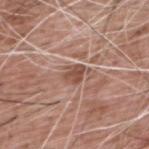Case summary:
– notes — catalogued during a skin exam; not biopsied
– image source — total-body-photography crop, ~15 mm field of view
– tile lighting — white-light illumination
– body site — the upper back
– lesion diameter — ~2.5 mm (longest diameter)
– automated metrics — a footprint of about 4 mm², an outline eccentricity of about 0.65 (0 = round, 1 = elongated), and a symmetry-axis asymmetry near 0.3; an average lesion color of about L≈50 a*≈21 b*≈27 (CIELAB), roughly 10 lightness units darker than nearby skin, and a normalized border contrast of about 7.5; a border-irregularity index near 3/10, internal color variation of about 2.5 on a 0–10 scale, and peripheral color asymmetry of about 1
– patient — male, aged around 60